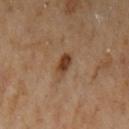biopsy_status: not biopsied; imaged during a skin examination
automated_metrics:
  area_mm2_approx: 4.0
  eccentricity: 0.85
  shape_asymmetry: 0.25
  vs_skin_darker_L: 12.0
  vs_skin_contrast_norm: 9.5
  nevus_likeness_0_100: 95
  lesion_detection_confidence_0_100: 100
image:
  source: total-body photography crop
  field_of_view_mm: 15
lighting: cross-polarized
patient:
  sex: female
  age_approx: 60
site: left upper arm
lesion_size:
  long_diameter_mm_approx: 3.0